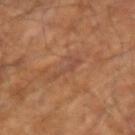Q: Was a biopsy performed?
A: catalogued during a skin exam; not biopsied